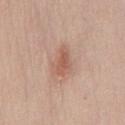{
  "biopsy_status": "not biopsied; imaged during a skin examination",
  "image": {
    "source": "total-body photography crop",
    "field_of_view_mm": 15
  },
  "site": "chest",
  "lesion_size": {
    "long_diameter_mm_approx": 3.5
  },
  "patient": {
    "sex": "male",
    "age_approx": 45
  }
}